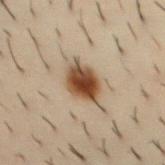Clinical impression:
Imaged during a routine full-body skin examination; the lesion was not biopsied and no histopathology is available.
Acquisition and patient details:
Automated tile analysis of the lesion measured a footprint of about 12 mm². The software also gave an average lesion color of about L≈39 a*≈14 b*≈27 (CIELAB). The analysis additionally found a border-irregularity rating of about 1.5/10, a within-lesion color-variation index near 7/10, and peripheral color asymmetry of about 2. And it measured a nevus-likeness score of about 100/100. A male patient in their 30s. The lesion's longest dimension is about 4.5 mm. The tile uses cross-polarized illumination. The lesion is located on the abdomen. This image is a 15 mm lesion crop taken from a total-body photograph.